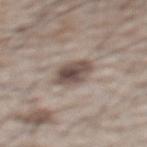Q: Is there a histopathology result?
A: imaged on a skin check; not biopsied
Q: Where on the body is the lesion?
A: the upper back
Q: Illumination type?
A: white-light illumination
Q: What did automated image analysis measure?
A: a lesion color around L≈49 a*≈13 b*≈20 in CIELAB, a lesion–skin lightness drop of about 14, and a lesion-to-skin contrast of about 10 (normalized; higher = more distinct)
Q: What is the imaging modality?
A: ~15 mm crop, total-body skin-cancer survey
Q: What are the patient's age and sex?
A: male, aged approximately 65